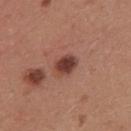Case summary:
- follow-up: no biopsy performed (imaged during a skin exam)
- illumination: white-light
- subject: female, aged 23–27
- acquisition: ~15 mm tile from a whole-body skin photo
- lesion diameter: ≈3 mm
- body site: the mid back
- automated metrics: a lesion area of about 5 mm²; a nevus-likeness score of about 90/100 and a lesion-detection confidence of about 100/100